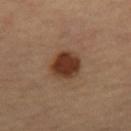{
  "biopsy_status": "not biopsied; imaged during a skin examination",
  "image": {
    "source": "total-body photography crop",
    "field_of_view_mm": 15
  },
  "patient": {
    "sex": "female",
    "age_approx": 70
  },
  "site": "right thigh",
  "lighting": "cross-polarized",
  "automated_metrics": {
    "area_mm2_approx": 11.0,
    "eccentricity": 0.6,
    "shape_asymmetry": 0.15,
    "border_irregularity_0_10": 1.5,
    "color_variation_0_10": 3.5,
    "peripheral_color_asymmetry": 1.0,
    "nevus_likeness_0_100": 100,
    "lesion_detection_confidence_0_100": 100
  }
}